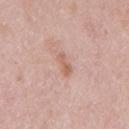The lesion was tiled from a total-body skin photograph and was not biopsied. The lesion's longest dimension is about 3 mm. A female subject, aged approximately 50. Located on the right upper arm. Cropped from a total-body skin-imaging series; the visible field is about 15 mm. This is a white-light tile.